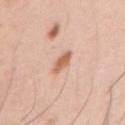Recorded during total-body skin imaging; not selected for excision or biopsy.
About 2.5 mm across.
A male subject aged 33 to 37.
Imaged with white-light lighting.
The lesion is on the right upper arm.
Cropped from a total-body skin-imaging series; the visible field is about 15 mm.